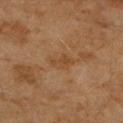Clinical impression:
Captured during whole-body skin photography for melanoma surveillance; the lesion was not biopsied.
Clinical summary:
A female subject aged 58 to 62. Located on the arm. About 3.5 mm across. Automated image analysis of the tile measured an average lesion color of about L≈42 a*≈18 b*≈33 (CIELAB) and a lesion-to-skin contrast of about 5 (normalized; higher = more distinct). The analysis additionally found a border-irregularity index near 3/10 and peripheral color asymmetry of about 0.5. It also reported an automated nevus-likeness rating near 0 out of 100. Cropped from a total-body skin-imaging series; the visible field is about 15 mm.